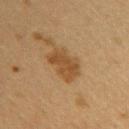Q: Is there a histopathology result?
A: catalogued during a skin exam; not biopsied
Q: Where on the body is the lesion?
A: the right upper arm
Q: Lesion size?
A: about 4.5 mm
Q: What is the imaging modality?
A: 15 mm crop, total-body photography
Q: How was the tile lit?
A: cross-polarized illumination
Q: What are the patient's age and sex?
A: female, aged 38–42